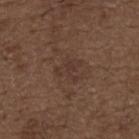Clinical summary: Cropped from a total-body skin-imaging series; the visible field is about 15 mm. Automated image analysis of the tile measured an area of roughly 5 mm², a shape eccentricity near 0.75, and two-axis asymmetry of about 0.55. The analysis additionally found a lesion color around L≈34 a*≈16 b*≈23 in CIELAB. It also reported border irregularity of about 7.5 on a 0–10 scale, internal color variation of about 1 on a 0–10 scale, and radial color variation of about 0.5. It also reported a classifier nevus-likeness of about 0/100. Located on the back. This is a white-light tile. Approximately 3.5 mm at its widest. A male patient about 50 years old.